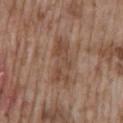Clinical impression: Part of a total-body skin-imaging series; this lesion was reviewed on a skin check and was not flagged for biopsy. Image and clinical context: A 15 mm close-up tile from a total-body photography series done for melanoma screening. From the mid back. About 6 mm across. A male patient approximately 75 years of age. Imaged with white-light lighting. The total-body-photography lesion software estimated a lesion area of about 9.5 mm², a shape eccentricity near 0.95, and two-axis asymmetry of about 0.45. The analysis additionally found border irregularity of about 7.5 on a 0–10 scale, a within-lesion color-variation index near 2.5/10, and radial color variation of about 0.5. The software also gave an automated nevus-likeness rating near 0 out of 100 and a detector confidence of about 100 out of 100 that the crop contains a lesion.